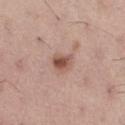Findings:
- biopsy status — imaged on a skin check; not biopsied
- anatomic site — the right thigh
- automated metrics — an area of roughly 4.5 mm², an eccentricity of roughly 0.7, and two-axis asymmetry of about 0.25; a mean CIELAB color near L≈52 a*≈21 b*≈26, about 12 CIELAB-L* units darker than the surrounding skin, and a lesion-to-skin contrast of about 8.5 (normalized; higher = more distinct); internal color variation of about 4.5 on a 0–10 scale and peripheral color asymmetry of about 1.5; a classifier nevus-likeness of about 90/100
- diameter — ≈2.5 mm
- acquisition — 15 mm crop, total-body photography
- illumination — white-light illumination
- subject — male, about 55 years old